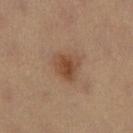Q: Was a biopsy performed?
A: imaged on a skin check; not biopsied
Q: Lesion location?
A: the right lower leg
Q: What is the imaging modality?
A: total-body-photography crop, ~15 mm field of view
Q: What are the patient's age and sex?
A: female, aged around 35